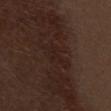notes: total-body-photography surveillance lesion; no biopsy
location: the front of the torso
imaging modality: total-body-photography crop, ~15 mm field of view
subject: male, aged approximately 70